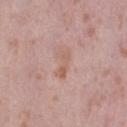follow-up = catalogued during a skin exam; not biopsied
diameter = ~3.5 mm (longest diameter)
site = the right thigh
illumination = white-light
image source = ~15 mm crop, total-body skin-cancer survey
automated metrics = a lesion color around L≈60 a*≈21 b*≈26 in CIELAB and a normalized lesion–skin contrast near 5.5
patient = female, aged 38–42